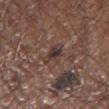Assessment:
This lesion was catalogued during total-body skin photography and was not selected for biopsy.
Acquisition and patient details:
This is a white-light tile. Located on the right upper arm. The lesion's longest dimension is about 3 mm. The patient is a male roughly 65 years of age. This image is a 15 mm lesion crop taken from a total-body photograph.